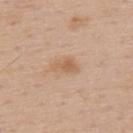The recorded lesion diameter is about 3.5 mm. Located on the back. A roughly 15 mm field-of-view crop from a total-body skin photograph. Automated tile analysis of the lesion measured an average lesion color of about L≈61 a*≈19 b*≈33 (CIELAB), a lesion–skin lightness drop of about 8, and a lesion-to-skin contrast of about 6 (normalized; higher = more distinct). The patient is a male in their mid-50s.